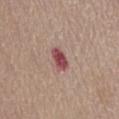The lesion is on the abdomen. A female patient, about 65 years old. A lesion tile, about 15 mm wide, cut from a 3D total-body photograph.